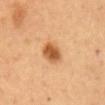Assessment:
This lesion was catalogued during total-body skin photography and was not selected for biopsy.
Image and clinical context:
Cropped from a total-body skin-imaging series; the visible field is about 15 mm. Automated tile analysis of the lesion measured a shape eccentricity near 0.55 and a symmetry-axis asymmetry near 0.1. The software also gave an automated nevus-likeness rating near 100 out of 100 and a detector confidence of about 100 out of 100 that the crop contains a lesion. A female patient. Located on the abdomen.